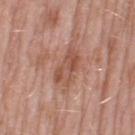– biopsy status · catalogued during a skin exam; not biopsied
– image source · ~15 mm tile from a whole-body skin photo
– lesion size · ~4.5 mm (longest diameter)
– location · the left thigh
– illumination · white-light illumination
– subject · female, about 70 years old